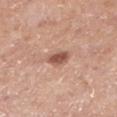The lesion was tiled from a total-body skin photograph and was not biopsied. The patient is a male roughly 55 years of age. Cropped from a total-body skin-imaging series; the visible field is about 15 mm. The lesion is located on the left lower leg.